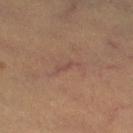Recorded during total-body skin imaging; not selected for excision or biopsy. The lesion's longest dimension is about 3 mm. A roughly 15 mm field-of-view crop from a total-body skin photograph. Imaged with cross-polarized lighting. A female patient, about 30 years old. The lesion is on the right thigh.